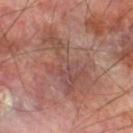Q: Was a biopsy performed?
A: catalogued during a skin exam; not biopsied
Q: Where on the body is the lesion?
A: the leg
Q: What kind of image is this?
A: ~15 mm crop, total-body skin-cancer survey
Q: Patient demographics?
A: male, approximately 70 years of age